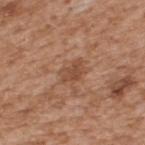biopsy status = total-body-photography surveillance lesion; no biopsy | patient = male, aged 63 to 67 | image = ~15 mm tile from a whole-body skin photo | location = the upper back | lighting = white-light | size = ~3 mm (longest diameter).